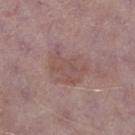follow-up=catalogued during a skin exam; not biopsied
acquisition=total-body-photography crop, ~15 mm field of view
subject=male, aged around 75
TBP lesion metrics=a lesion area of about 13 mm², a shape eccentricity near 0.65, and a symmetry-axis asymmetry near 0.35; a mean CIELAB color near L≈51 a*≈19 b*≈21, roughly 6 lightness units darker than nearby skin, and a normalized border contrast of about 5; an automated nevus-likeness rating near 0 out of 100 and a lesion-detection confidence of about 100/100
lighting=white-light illumination
site=the right lower leg